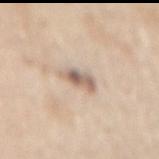No biopsy was performed on this lesion — it was imaged during a full skin examination and was not determined to be concerning. Longest diameter approximately 3.5 mm. A roughly 15 mm field-of-view crop from a total-body skin photograph. The subject is a female about 65 years old. The tile uses white-light illumination. Located on the mid back.